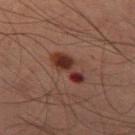The lesion was photographed on a routine skin check and not biopsied; there is no pathology result. Longest diameter approximately 4.5 mm. On the left thigh. Imaged with cross-polarized lighting. A 15 mm close-up extracted from a 3D total-body photography capture. A male subject about 60 years old.